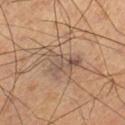The lesion was tiled from a total-body skin photograph and was not biopsied.
Cropped from a total-body skin-imaging series; the visible field is about 15 mm.
The patient is a male roughly 60 years of age.
About 4.5 mm across.
Located on the right lower leg.
An algorithmic analysis of the crop reported a shape eccentricity near 0.85 and two-axis asymmetry of about 0.7. The software also gave an average lesion color of about L≈51 a*≈15 b*≈25 (CIELAB) and a normalized lesion–skin contrast near 7. And it measured a border-irregularity index near 8.5/10 and a peripheral color-asymmetry measure near 1.5.